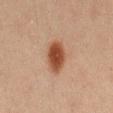biopsy_status: not biopsied; imaged during a skin examination
image:
  source: total-body photography crop
  field_of_view_mm: 15
automated_metrics:
  area_mm2_approx: 9.0
  eccentricity: 0.75
  shape_asymmetry: 0.2
  cielab_L: 42
  cielab_a: 22
  cielab_b: 30
  vs_skin_darker_L: 13.0
  color_variation_0_10: 3.5
  peripheral_color_asymmetry: 1.0
  nevus_likeness_0_100: 100
lesion_size:
  long_diameter_mm_approx: 4.0
site: leg
patient:
  sex: female
  age_approx: 20
lighting: cross-polarized